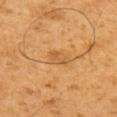The lesion is located on the left upper arm. A male subject about 60 years old. A lesion tile, about 15 mm wide, cut from a 3D total-body photograph.